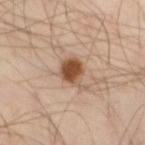| field | value |
|---|---|
| workup | catalogued during a skin exam; not biopsied |
| tile lighting | cross-polarized |
| lesion size | ≈4.5 mm |
| acquisition | ~15 mm tile from a whole-body skin photo |
| TBP lesion metrics | border irregularity of about 4.5 on a 0–10 scale, a color-variation rating of about 5/10, and radial color variation of about 1.5; an automated nevus-likeness rating near 100 out of 100 and lesion-presence confidence of about 100/100 |
| body site | the right thigh |
| subject | male, aged approximately 45 |